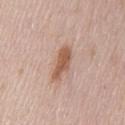Captured during whole-body skin photography for melanoma surveillance; the lesion was not biopsied.
A region of skin cropped from a whole-body photographic capture, roughly 15 mm wide.
The lesion is located on the mid back.
A male patient, in their mid-60s.
The lesion's longest dimension is about 4.5 mm.